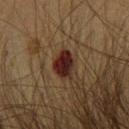No biopsy was performed on this lesion — it was imaged during a full skin examination and was not determined to be concerning. From the chest. Measured at roughly 3.5 mm in maximum diameter. This is a cross-polarized tile. A 15 mm crop from a total-body photograph taken for skin-cancer surveillance. A male patient approximately 50 years of age.